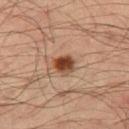follow-up — no biopsy performed (imaged during a skin exam)
patient — male, aged approximately 35
image-analysis metrics — a footprint of about 5 mm² and an eccentricity of roughly 0.4; a lesion–skin lightness drop of about 15 and a normalized lesion–skin contrast near 12; a border-irregularity rating of about 1.5/10; a nevus-likeness score of about 100/100 and a lesion-detection confidence of about 100/100
lesion diameter — about 2.5 mm
lighting — cross-polarized illumination
site — the left thigh
imaging modality — 15 mm crop, total-body photography